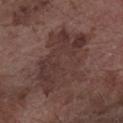No biopsy was performed on this lesion — it was imaged during a full skin examination and was not determined to be concerning.
On the right forearm.
Automated tile analysis of the lesion measured a lesion area of about 33 mm², a shape eccentricity near 0.8, and a symmetry-axis asymmetry near 0.3. And it measured an automated nevus-likeness rating near 0 out of 100 and lesion-presence confidence of about 100/100.
Measured at roughly 8.5 mm in maximum diameter.
Cropped from a total-body skin-imaging series; the visible field is about 15 mm.
A male subject, aged approximately 75.
Imaged with white-light lighting.